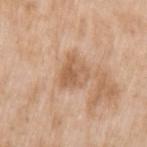follow-up: no biopsy performed (imaged during a skin exam)
location: the arm
image source: 15 mm crop, total-body photography
patient: female, aged around 75